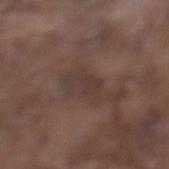notes = no biopsy performed (imaged during a skin exam)
patient = male, aged 78–82
anatomic site = the left lower leg
image-analysis metrics = a footprint of about 6 mm² and two-axis asymmetry of about 0.4; about 6 CIELAB-L* units darker than the surrounding skin and a normalized border contrast of about 5.5; a border-irregularity index near 5/10, internal color variation of about 2 on a 0–10 scale, and peripheral color asymmetry of about 1
image source = 15 mm crop, total-body photography
size = about 4 mm